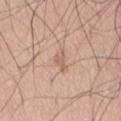| feature | finding |
|---|---|
| follow-up | catalogued during a skin exam; not biopsied |
| image | 15 mm crop, total-body photography |
| site | the abdomen |
| subject | male, about 40 years old |